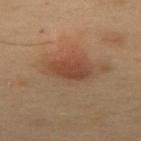This lesion was catalogued during total-body skin photography and was not selected for biopsy. The lesion's longest dimension is about 3.5 mm. The patient is a male aged 53 to 57. A 15 mm crop from a total-body photograph taken for skin-cancer surveillance. The total-body-photography lesion software estimated roughly 7 lightness units darker than nearby skin and a normalized border contrast of about 7. And it measured border irregularity of about 1.5 on a 0–10 scale, a within-lesion color-variation index near 2.5/10, and a peripheral color-asymmetry measure near 1. From the abdomen.